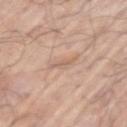| key | value |
|---|---|
| workup | total-body-photography surveillance lesion; no biopsy |
| imaging modality | ~15 mm tile from a whole-body skin photo |
| subject | male, approximately 70 years of age |
| lighting | white-light |
| body site | the left upper arm |